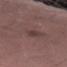The lesion was tiled from a total-body skin photograph and was not biopsied. The lesion-visualizer software estimated a lesion area of about 4 mm² and a symmetry-axis asymmetry near 0.2. A roughly 15 mm field-of-view crop from a total-body skin photograph. Located on the right thigh. About 3 mm across. The tile uses white-light illumination. The subject is a male about 55 years old.